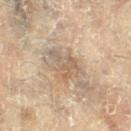workup: total-body-photography surveillance lesion; no biopsy
image-analysis metrics: a footprint of about 8 mm², an eccentricity of roughly 0.7, and a shape-asymmetry score of about 0.5 (0 = symmetric); a mean CIELAB color near L≈54 a*≈12 b*≈27, roughly 8 lightness units darker than nearby skin, and a normalized lesion–skin contrast near 5.5; border irregularity of about 5.5 on a 0–10 scale and a peripheral color-asymmetry measure near 1.5; an automated nevus-likeness rating near 0 out of 100
anatomic site: the right leg
patient: female, approximately 80 years of age
image source: 15 mm crop, total-body photography
lesion diameter: about 4 mm